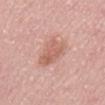Impression:
Part of a total-body skin-imaging series; this lesion was reviewed on a skin check and was not flagged for biopsy.
Background:
The lesion is located on the abdomen. A region of skin cropped from a whole-body photographic capture, roughly 15 mm wide. The tile uses white-light illumination. The total-body-photography lesion software estimated a lesion color around L≈62 a*≈23 b*≈28 in CIELAB and a normalized lesion–skin contrast near 6. The patient is a male aged 53 to 57. Longest diameter approximately 4.5 mm.